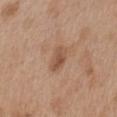Clinical impression:
No biopsy was performed on this lesion — it was imaged during a full skin examination and was not determined to be concerning.
Clinical summary:
This image is a 15 mm lesion crop taken from a total-body photograph. The lesion is located on the chest. A male subject about 55 years old.